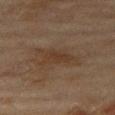Imaged during a routine full-body skin examination; the lesion was not biopsied and no histopathology is available.
Located on the right thigh.
The patient is a female aged 58–62.
Captured under cross-polarized illumination.
The recorded lesion diameter is about 4.5 mm.
Automated image analysis of the tile measured a classifier nevus-likeness of about 0/100 and a lesion-detection confidence of about 100/100.
Cropped from a total-body skin-imaging series; the visible field is about 15 mm.